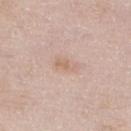{"biopsy_status": "not biopsied; imaged during a skin examination", "lesion_size": {"long_diameter_mm_approx": 3.0}, "image": {"source": "total-body photography crop", "field_of_view_mm": 15}, "site": "right lower leg", "patient": {"sex": "male", "age_approx": 80}, "lighting": "white-light"}